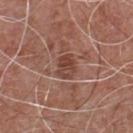Cropped from a total-body skin-imaging series; the visible field is about 15 mm. The lesion-visualizer software estimated an eccentricity of roughly 0.65 and a shape-asymmetry score of about 0.25 (0 = symmetric). The software also gave an average lesion color of about L≈44 a*≈22 b*≈26 (CIELAB), about 9 CIELAB-L* units darker than the surrounding skin, and a lesion-to-skin contrast of about 7 (normalized; higher = more distinct). The analysis additionally found an automated nevus-likeness rating near 0 out of 100. A male patient approximately 55 years of age. The lesion's longest dimension is about 3 mm. The lesion is on the front of the torso.